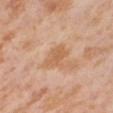  biopsy_status: not biopsied; imaged during a skin examination
  lighting: cross-polarized
  lesion_size:
    long_diameter_mm_approx: 3.0
  patient:
    sex: female
    age_approx: 55
  site: leg
  image:
    source: total-body photography crop
    field_of_view_mm: 15